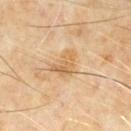* notes — catalogued during a skin exam; not biopsied
* lighting — cross-polarized illumination
* site — the mid back
* image source — 15 mm crop, total-body photography
* lesion diameter — ~3.5 mm (longest diameter)
* subject — male, in their 60s
* automated lesion analysis — an average lesion color of about L≈61 a*≈17 b*≈39 (CIELAB) and a normalized border contrast of about 6.5; a peripheral color-asymmetry measure near 1